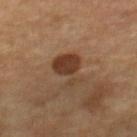Clinical impression:
Part of a total-body skin-imaging series; this lesion was reviewed on a skin check and was not flagged for biopsy.
Acquisition and patient details:
On the right lower leg. The tile uses cross-polarized illumination. A 15 mm close-up tile from a total-body photography series done for melanoma screening. The lesion's longest dimension is about 4.5 mm. A female patient, aged around 70.